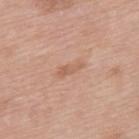Impression:
No biopsy was performed on this lesion — it was imaged during a full skin examination and was not determined to be concerning.
Acquisition and patient details:
Longest diameter approximately 3.5 mm. The tile uses white-light illumination. The total-body-photography lesion software estimated a lesion area of about 3.5 mm², a shape eccentricity near 0.95, and two-axis asymmetry of about 0.4. It also reported border irregularity of about 4 on a 0–10 scale, internal color variation of about 0 on a 0–10 scale, and radial color variation of about 0. A lesion tile, about 15 mm wide, cut from a 3D total-body photograph. A female subject, aged around 60. On the upper back.